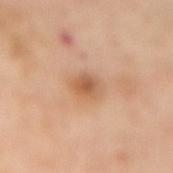follow-up=no biopsy performed (imaged during a skin exam) | diameter=about 3 mm | tile lighting=cross-polarized illumination | image source=total-body-photography crop, ~15 mm field of view | site=the mid back | patient=female, approximately 55 years of age.